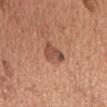A lesion tile, about 15 mm wide, cut from a 3D total-body photograph.
Automated image analysis of the tile measured an area of roughly 5.5 mm², an eccentricity of roughly 0.6, and two-axis asymmetry of about 0.4. The analysis additionally found internal color variation of about 4.5 on a 0–10 scale and radial color variation of about 1.5. And it measured a nevus-likeness score of about 50/100 and lesion-presence confidence of about 100/100.
Captured under white-light illumination.
On the chest.
Measured at roughly 3.5 mm in maximum diameter.
A male subject, in their mid- to late 60s.